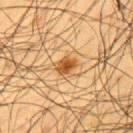Q: Was this lesion biopsied?
A: no biopsy performed (imaged during a skin exam)
Q: How was this image acquired?
A: ~15 mm crop, total-body skin-cancer survey
Q: Where on the body is the lesion?
A: the upper back
Q: Patient demographics?
A: male, in their 60s